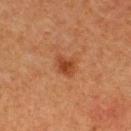The lesion was tiled from a total-body skin photograph and was not biopsied. A close-up tile cropped from a whole-body skin photograph, about 15 mm across. Automated image analysis of the tile measured a footprint of about 4.5 mm², an outline eccentricity of about 0.6 (0 = round, 1 = elongated), and two-axis asymmetry of about 0.25. It also reported a classifier nevus-likeness of about 80/100. Approximately 2.5 mm at its widest. The lesion is located on the upper back. Captured under cross-polarized illumination. A female patient aged 38 to 42.